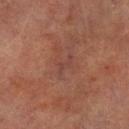No biopsy was performed on this lesion — it was imaged during a full skin examination and was not determined to be concerning. Captured under cross-polarized illumination. The patient is a male in their 70s. Cropped from a total-body skin-imaging series; the visible field is about 15 mm. Located on the right lower leg.